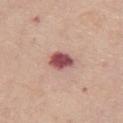The lesion was photographed on a routine skin check and not biopsied; there is no pathology result. Automated image analysis of the tile measured a footprint of about 6.5 mm², an outline eccentricity of about 0.7 (0 = round, 1 = elongated), and a shape-asymmetry score of about 0.2 (0 = symmetric). And it measured a lesion color around L≈52 a*≈27 b*≈21 in CIELAB and a lesion–skin lightness drop of about 17. The lesion's longest dimension is about 3.5 mm. From the chest. A female patient, roughly 70 years of age. Captured under white-light illumination. This image is a 15 mm lesion crop taken from a total-body photograph.